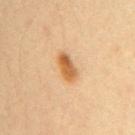Notes:
– patient — female, aged 28–32
– automated metrics — an area of roughly 6 mm², an outline eccentricity of about 0.85 (0 = round, 1 = elongated), and a symmetry-axis asymmetry near 0.15; a lesion–skin lightness drop of about 12 and a normalized lesion–skin contrast near 9; a border-irregularity rating of about 2/10, a color-variation rating of about 4.5/10, and a peripheral color-asymmetry measure near 1.5; a classifier nevus-likeness of about 95/100 and lesion-presence confidence of about 100/100
– image — 15 mm crop, total-body photography
– diameter — ~3.5 mm (longest diameter)
– site — the right upper arm
– illumination — cross-polarized illumination